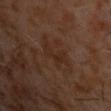Recorded during total-body skin imaging; not selected for excision or biopsy.
Measured at roughly 3.5 mm in maximum diameter.
A 15 mm close-up tile from a total-body photography series done for melanoma screening.
On the chest.
The patient is a male about 60 years old.